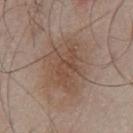Part of a total-body skin-imaging series; this lesion was reviewed on a skin check and was not flagged for biopsy. The lesion is located on the chest. Automated tile analysis of the lesion measured a lesion–skin lightness drop of about 8. Cropped from a whole-body photographic skin survey; the tile spans about 15 mm. This is a white-light tile. The subject is a male aged 63–67.